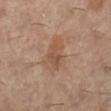Background:
The tile uses cross-polarized illumination. The lesion is located on the left lower leg. A 15 mm close-up tile from a total-body photography series done for melanoma screening. An algorithmic analysis of the crop reported a mean CIELAB color near L≈52 a*≈19 b*≈31, a lesion–skin lightness drop of about 7, and a normalized border contrast of about 6. The analysis additionally found an automated nevus-likeness rating near 10 out of 100. A female subject in their 60s.